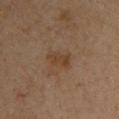Imaged during a routine full-body skin examination; the lesion was not biopsied and no histopathology is available. The tile uses cross-polarized illumination. Longest diameter approximately 3.5 mm. On the chest. The subject is a male approximately 35 years of age. A 15 mm close-up tile from a total-body photography series done for melanoma screening.